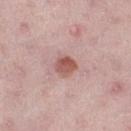Clinical impression: Captured during whole-body skin photography for melanoma surveillance; the lesion was not biopsied. Clinical summary: On the left thigh. A female patient, aged 43–47. This is a white-light tile. A lesion tile, about 15 mm wide, cut from a 3D total-body photograph.